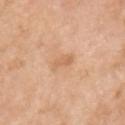Q: Was this lesion biopsied?
A: no biopsy performed (imaged during a skin exam)
Q: What did automated image analysis measure?
A: a lesion area of about 3.5 mm²; roughly 8 lightness units darker than nearby skin; border irregularity of about 3 on a 0–10 scale and internal color variation of about 0.5 on a 0–10 scale; a classifier nevus-likeness of about 0/100 and a lesion-detection confidence of about 100/100
Q: How was the tile lit?
A: white-light
Q: What is the imaging modality?
A: ~15 mm crop, total-body skin-cancer survey
Q: Patient demographics?
A: male, roughly 50 years of age
Q: What is the anatomic site?
A: the upper back